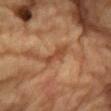A male patient aged approximately 85 or older.
On the front of the torso.
Automated tile analysis of the lesion measured a lesion color around L≈40 a*≈20 b*≈31 in CIELAB, a lesion–skin lightness drop of about 8, and a normalized lesion–skin contrast near 7.
A region of skin cropped from a whole-body photographic capture, roughly 15 mm wide.
The lesion's longest dimension is about 4 mm.